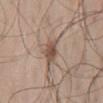notes = no biopsy performed (imaged during a skin exam) | lighting = white-light | TBP lesion metrics = an average lesion color of about L≈51 a*≈16 b*≈26 (CIELAB), about 11 CIELAB-L* units darker than the surrounding skin, and a normalized lesion–skin contrast near 8; a nevus-likeness score of about 70/100 | size = about 3.5 mm | patient = male, aged around 45 | acquisition = ~15 mm crop, total-body skin-cancer survey | anatomic site = the mid back.